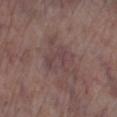No biopsy was performed on this lesion — it was imaged during a full skin examination and was not determined to be concerning. Imaged with white-light lighting. A lesion tile, about 15 mm wide, cut from a 3D total-body photograph. The lesion is located on the leg. Longest diameter approximately 3.5 mm. Automated tile analysis of the lesion measured a lesion area of about 7.5 mm² and a symmetry-axis asymmetry near 0.25. It also reported a mean CIELAB color near L≈42 a*≈17 b*≈16 and a lesion–skin lightness drop of about 5. The analysis additionally found border irregularity of about 3 on a 0–10 scale and peripheral color asymmetry of about 1. And it measured a nevus-likeness score of about 0/100. The patient is a male aged approximately 70.